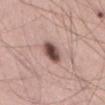Imaged during a routine full-body skin examination; the lesion was not biopsied and no histopathology is available. The tile uses white-light illumination. A lesion tile, about 15 mm wide, cut from a 3D total-body photograph. A male patient, roughly 50 years of age. Automated tile analysis of the lesion measured a mean CIELAB color near L≈48 a*≈19 b*≈21, roughly 19 lightness units darker than nearby skin, and a lesion-to-skin contrast of about 12.5 (normalized; higher = more distinct). The analysis additionally found an automated nevus-likeness rating near 100 out of 100. The lesion is on the abdomen.